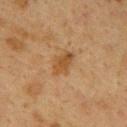workup = imaged on a skin check; not biopsied
tile lighting = cross-polarized illumination
subject = female, aged approximately 40
imaging modality = 15 mm crop, total-body photography
diameter = about 3.5 mm
location = the upper back